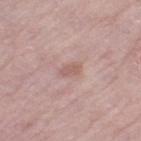Assessment:
The lesion was tiled from a total-body skin photograph and was not biopsied.
Context:
The lesion is located on the leg. Automated image analysis of the tile measured a lesion color around L≈58 a*≈20 b*≈23 in CIELAB and about 8 CIELAB-L* units darker than the surrounding skin. The analysis additionally found lesion-presence confidence of about 100/100. A 15 mm crop from a total-body photograph taken for skin-cancer surveillance. A female subject aged 68 to 72.